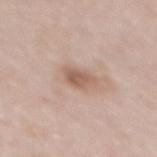Q: Lesion size?
A: ≈3 mm
Q: Patient demographics?
A: female, roughly 30 years of age
Q: How was this image acquired?
A: ~15 mm tile from a whole-body skin photo
Q: Lesion location?
A: the mid back
Q: Illumination type?
A: white-light